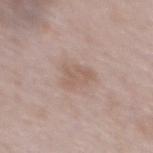Assessment:
Recorded during total-body skin imaging; not selected for excision or biopsy.
Context:
Located on the upper back. The subject is a female about 65 years old. Cropped from a total-body skin-imaging series; the visible field is about 15 mm. This is a white-light tile.